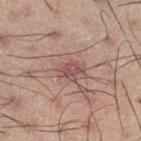No biopsy was performed on this lesion — it was imaged during a full skin examination and was not determined to be concerning. The lesion-visualizer software estimated a border-irregularity rating of about 3/10 and a within-lesion color-variation index near 4/10. The analysis additionally found an automated nevus-likeness rating near 0 out of 100 and lesion-presence confidence of about 100/100. This is a white-light tile. A male subject, aged around 55. The lesion's longest dimension is about 3 mm. From the right thigh. A 15 mm close-up tile from a total-body photography series done for melanoma screening.